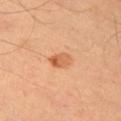Q: Was a biopsy performed?
A: no biopsy performed (imaged during a skin exam)
Q: How was this image acquired?
A: total-body-photography crop, ~15 mm field of view
Q: Lesion location?
A: the right upper arm
Q: What are the patient's age and sex?
A: male, approximately 45 years of age